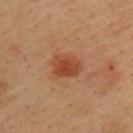The lesion was tiled from a total-body skin photograph and was not biopsied. The lesion's longest dimension is about 3.5 mm. From the upper back. This is a cross-polarized tile. Automated image analysis of the tile measured a shape eccentricity near 0.65. The software also gave a mean CIELAB color near L≈46 a*≈27 b*≈36, roughly 10 lightness units darker than nearby skin, and a lesion-to-skin contrast of about 8 (normalized; higher = more distinct). The patient is a male aged approximately 40. A 15 mm close-up tile from a total-body photography series done for melanoma screening.